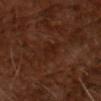workup: imaged on a skin check; not biopsied
imaging modality: 15 mm crop, total-body photography
lighting: cross-polarized illumination
diameter: ≈3.5 mm
patient: male, in their mid-60s